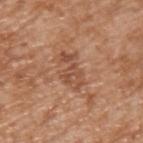Impression: The lesion was photographed on a routine skin check and not biopsied; there is no pathology result. Image and clinical context: Cropped from a total-body skin-imaging series; the visible field is about 15 mm. The patient is a male about 65 years old. This is a white-light tile. The recorded lesion diameter is about 4.5 mm. The lesion is on the left upper arm. The lesion-visualizer software estimated an outline eccentricity of about 0.8 (0 = round, 1 = elongated). The software also gave a border-irregularity rating of about 5.5/10, internal color variation of about 3.5 on a 0–10 scale, and peripheral color asymmetry of about 1. The software also gave a classifier nevus-likeness of about 5/100 and a detector confidence of about 100 out of 100 that the crop contains a lesion.